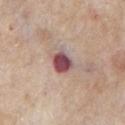<tbp_lesion>
  <image>
    <source>total-body photography crop</source>
    <field_of_view_mm>15</field_of_view_mm>
  </image>
  <patient>
    <sex>male</sex>
    <age_approx>65</age_approx>
  </patient>
  <automated_metrics>
    <cielab_L>49</cielab_L>
    <cielab_a>24</cielab_a>
    <cielab_b>18</cielab_b>
    <vs_skin_darker_L>18.0</vs_skin_darker_L>
    <vs_skin_contrast_norm>12.5</vs_skin_contrast_norm>
    <peripheral_color_asymmetry>1.5</peripheral_color_asymmetry>
  </automated_metrics>
  <lighting>white-light</lighting>
  <site>chest</site>
  <lesion_size>
    <long_diameter_mm_approx>3.0</long_diameter_mm_approx>
  </lesion_size>
</tbp_lesion>